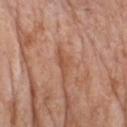Impression: Recorded during total-body skin imaging; not selected for excision or biopsy. Background: The total-body-photography lesion software estimated an area of roughly 3 mm², an outline eccentricity of about 0.95 (0 = round, 1 = elongated), and two-axis asymmetry of about 0.35. The analysis additionally found a color-variation rating of about 0.5/10 and peripheral color asymmetry of about 0. The recorded lesion diameter is about 3.5 mm. A region of skin cropped from a whole-body photographic capture, roughly 15 mm wide. On the chest. Captured under white-light illumination. The subject is a female roughly 70 years of age.